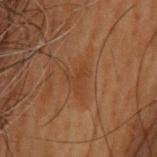Assessment: This lesion was catalogued during total-body skin photography and was not selected for biopsy. Context: On the upper back. This image is a 15 mm lesion crop taken from a total-body photograph. Approximately 3 mm at its widest. The patient is a male roughly 55 years of age.